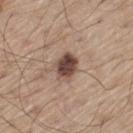follow-up: imaged on a skin check; not biopsied | anatomic site: the left thigh | size: ≈3.5 mm | image-analysis metrics: border irregularity of about 2 on a 0–10 scale and a color-variation rating of about 6/10 | patient: male, aged around 60 | image: 15 mm crop, total-body photography | lighting: white-light illumination.